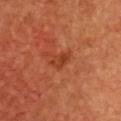Q: Was this lesion biopsied?
A: catalogued during a skin exam; not biopsied
Q: What kind of image is this?
A: total-body-photography crop, ~15 mm field of view
Q: Lesion size?
A: ~2.5 mm (longest diameter)
Q: What are the patient's age and sex?
A: female, roughly 45 years of age
Q: What is the anatomic site?
A: the chest
Q: How was the tile lit?
A: cross-polarized illumination
Q: What did automated image analysis measure?
A: an outline eccentricity of about 0.75 (0 = round, 1 = elongated) and a shape-asymmetry score of about 0.25 (0 = symmetric); a lesion color around L≈41 a*≈32 b*≈38 in CIELAB, about 7 CIELAB-L* units darker than the surrounding skin, and a normalized lesion–skin contrast near 6; a classifier nevus-likeness of about 0/100 and a detector confidence of about 100 out of 100 that the crop contains a lesion